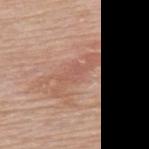This lesion was catalogued during total-body skin photography and was not selected for biopsy. The lesion is located on the back. Measured at roughly 6.5 mm in maximum diameter. This is a white-light tile. Cropped from a whole-body photographic skin survey; the tile spans about 15 mm. A male patient aged approximately 80.